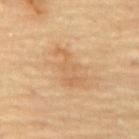Notes:
• biopsy status: imaged on a skin check; not biopsied
• imaging modality: ~15 mm tile from a whole-body skin photo
• tile lighting: cross-polarized illumination
• location: the mid back
• diameter: about 5 mm
• TBP lesion metrics: a lesion area of about 7.5 mm², an outline eccentricity of about 0.9 (0 = round, 1 = elongated), and a symmetry-axis asymmetry near 0.45; a nevus-likeness score of about 0/100
• patient: male, aged around 85 or older